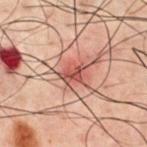Clinical impression: Recorded during total-body skin imaging; not selected for excision or biopsy. Acquisition and patient details: About 2.5 mm across. The lesion is located on the front of the torso. Automated tile analysis of the lesion measured a mean CIELAB color near L≈39 a*≈23 b*≈23 and a normalized lesion–skin contrast near 8.5. It also reported a classifier nevus-likeness of about 15/100 and a detector confidence of about 100 out of 100 that the crop contains a lesion. The tile uses cross-polarized illumination. A lesion tile, about 15 mm wide, cut from a 3D total-body photograph. A male patient approximately 60 years of age.